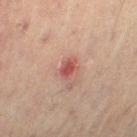Q: What are the patient's age and sex?
A: male, aged 58 to 62
Q: What is the anatomic site?
A: the leg
Q: How was the tile lit?
A: cross-polarized illumination
Q: What is the imaging modality?
A: 15 mm crop, total-body photography